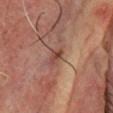subject: male, aged around 75
anatomic site: the head or neck
acquisition: ~15 mm tile from a whole-body skin photo